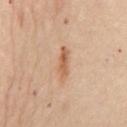The lesion was tiled from a total-body skin photograph and was not biopsied.
Automated tile analysis of the lesion measured a lesion color around L≈59 a*≈22 b*≈35 in CIELAB, a lesion–skin lightness drop of about 10, and a normalized border contrast of about 7.5. And it measured border irregularity of about 4 on a 0–10 scale, a within-lesion color-variation index near 3/10, and a peripheral color-asymmetry measure near 1. The software also gave a detector confidence of about 100 out of 100 that the crop contains a lesion.
The recorded lesion diameter is about 4 mm.
A 15 mm crop from a total-body photograph taken for skin-cancer surveillance.
The lesion is located on the mid back.
Imaged with cross-polarized lighting.
A female patient aged approximately 60.